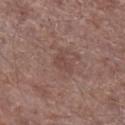Recorded during total-body skin imaging; not selected for excision or biopsy.
From the right lower leg.
A male patient aged 68 to 72.
A roughly 15 mm field-of-view crop from a total-body skin photograph.